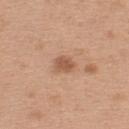- biopsy status: total-body-photography surveillance lesion; no biopsy
- size: ≈2.5 mm
- image source: 15 mm crop, total-body photography
- image-analysis metrics: a lesion area of about 4 mm², an eccentricity of roughly 0.65, and two-axis asymmetry of about 0.15; a lesion color around L≈55 a*≈22 b*≈32 in CIELAB, roughly 10 lightness units darker than nearby skin, and a lesion-to-skin contrast of about 7 (normalized; higher = more distinct); border irregularity of about 1.5 on a 0–10 scale, a color-variation rating of about 2/10, and a peripheral color-asymmetry measure near 1; lesion-presence confidence of about 100/100
- location: the back
- subject: female, aged 38 to 42